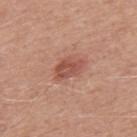– follow-up · imaged on a skin check; not biopsied
– imaging modality · 15 mm crop, total-body photography
– site · the upper back
– patient · male, aged 48–52
– lesion diameter · about 3.5 mm